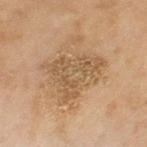The lesion was tiled from a total-body skin photograph and was not biopsied. An algorithmic analysis of the crop reported a lesion area of about 16 mm², an outline eccentricity of about 0.7 (0 = round, 1 = elongated), and two-axis asymmetry of about 0.55. And it measured roughly 8 lightness units darker than nearby skin and a normalized border contrast of about 6. From the left thigh. A female patient aged 58–62. The tile uses cross-polarized illumination. The lesion's longest dimension is about 6 mm. A region of skin cropped from a whole-body photographic capture, roughly 15 mm wide.